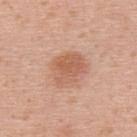Impression:
Part of a total-body skin-imaging series; this lesion was reviewed on a skin check and was not flagged for biopsy.
Background:
Located on the upper back. Approximately 4 mm at its widest. Captured under white-light illumination. A female patient, approximately 30 years of age. A roughly 15 mm field-of-view crop from a total-body skin photograph.